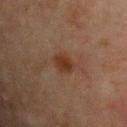Image and clinical context: The lesion-visualizer software estimated border irregularity of about 1.5 on a 0–10 scale, internal color variation of about 2.5 on a 0–10 scale, and radial color variation of about 1. A close-up tile cropped from a whole-body skin photograph, about 15 mm across. The recorded lesion diameter is about 3 mm. On the chest. The tile uses cross-polarized illumination. The subject is a male aged around 65.